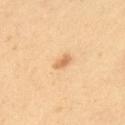Clinical impression: This lesion was catalogued during total-body skin photography and was not selected for biopsy. Background: The lesion is located on the upper back. A male patient, aged 38–42. The total-body-photography lesion software estimated a shape eccentricity near 0.85 and a symmetry-axis asymmetry near 0.3. And it measured a within-lesion color-variation index near 0/10. The software also gave an automated nevus-likeness rating near 80 out of 100 and a detector confidence of about 100 out of 100 that the crop contains a lesion. A region of skin cropped from a whole-body photographic capture, roughly 15 mm wide. About 2.5 mm across. The tile uses cross-polarized illumination.